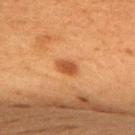follow-up: catalogued during a skin exam; not biopsied | anatomic site: the back | acquisition: ~15 mm crop, total-body skin-cancer survey | automated metrics: a footprint of about 3.5 mm², an outline eccentricity of about 0.75 (0 = round, 1 = elongated), and a shape-asymmetry score of about 0.25 (0 = symmetric) | tile lighting: cross-polarized illumination | subject: female, aged around 50 | diameter: about 2.5 mm.